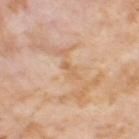biopsy status = no biopsy performed (imaged during a skin exam).